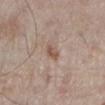Notes:
- notes · imaged on a skin check; not biopsied
- illumination · white-light
- subject · male, approximately 60 years of age
- image · ~15 mm crop, total-body skin-cancer survey
- image-analysis metrics · a footprint of about 3 mm², an outline eccentricity of about 0.9 (0 = round, 1 = elongated), and a shape-asymmetry score of about 0.3 (0 = symmetric); a mean CIELAB color near L≈54 a*≈17 b*≈26, roughly 9 lightness units darker than nearby skin, and a normalized border contrast of about 7; a border-irregularity index near 3/10, a within-lesion color-variation index near 1.5/10, and radial color variation of about 0.5
- body site · the leg